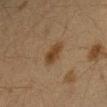No biopsy was performed on this lesion — it was imaged during a full skin examination and was not determined to be concerning.
Cropped from a total-body skin-imaging series; the visible field is about 15 mm.
This is a cross-polarized tile.
The subject is a female approximately 40 years of age.
From the left forearm.
Longest diameter approximately 3 mm.